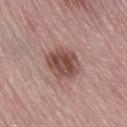Recorded during total-body skin imaging; not selected for excision or biopsy. A 15 mm close-up tile from a total-body photography series done for melanoma screening. Longest diameter approximately 4 mm. Captured under white-light illumination. An algorithmic analysis of the crop reported an area of roughly 12 mm² and a shape eccentricity near 0.55. It also reported a mean CIELAB color near L≈48 a*≈21 b*≈23, about 13 CIELAB-L* units darker than the surrounding skin, and a normalized border contrast of about 9.5. And it measured a color-variation rating of about 5.5/10 and peripheral color asymmetry of about 2. From the right thigh. A female patient, in their mid-60s.